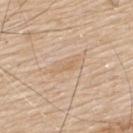{
  "biopsy_status": "not biopsied; imaged during a skin examination",
  "site": "upper back",
  "lesion_size": {
    "long_diameter_mm_approx": 2.5
  },
  "image": {
    "source": "total-body photography crop",
    "field_of_view_mm": 15
  },
  "patient": {
    "sex": "male",
    "age_approx": 80
  }
}